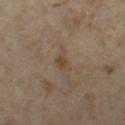Clinical impression: Captured during whole-body skin photography for melanoma surveillance; the lesion was not biopsied. Background: A lesion tile, about 15 mm wide, cut from a 3D total-body photograph. Automated tile analysis of the lesion measured a shape eccentricity near 0.75 and a symmetry-axis asymmetry near 0.4. The software also gave a border-irregularity index near 4/10 and radial color variation of about 0.5. The analysis additionally found an automated nevus-likeness rating near 0 out of 100 and a lesion-detection confidence of about 100/100. The lesion's longest dimension is about 2.5 mm. From the left thigh. The subject is a female in their mid-30s. This is a cross-polarized tile.